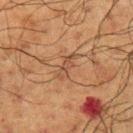The lesion was photographed on a routine skin check and not biopsied; there is no pathology result. A male patient, aged 58–62. The lesion's longest dimension is about 3 mm. The lesion is located on the left upper arm. This is a cross-polarized tile. This image is a 15 mm lesion crop taken from a total-body photograph.